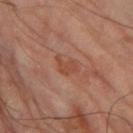Imaged during a routine full-body skin examination; the lesion was not biopsied and no histopathology is available. Automated image analysis of the tile measured a lesion area of about 4 mm², a shape eccentricity near 0.7, and a symmetry-axis asymmetry near 0.35. And it measured a lesion color around L≈47 a*≈24 b*≈31 in CIELAB, a lesion–skin lightness drop of about 7, and a normalized lesion–skin contrast near 6. The tile uses cross-polarized illumination. A close-up tile cropped from a whole-body skin photograph, about 15 mm across. From the left thigh. A male subject, about 65 years old. The lesion's longest dimension is about 3 mm.